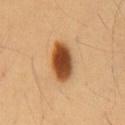No biopsy was performed on this lesion — it was imaged during a full skin examination and was not determined to be concerning. The lesion is on the chest. Imaged with cross-polarized lighting. Cropped from a total-body skin-imaging series; the visible field is about 15 mm. The subject is a male approximately 55 years of age.